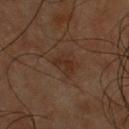  biopsy_status: not biopsied; imaged during a skin examination
  patient:
    sex: male
    age_approx: 60
  automated_metrics:
    area_mm2_approx: 5.0
    eccentricity: 0.65
    shape_asymmetry: 0.4
    border_irregularity_0_10: 3.5
    color_variation_0_10: 2.5
    peripheral_color_asymmetry: 1.0
  image:
    source: total-body photography crop
    field_of_view_mm: 15
  lesion_size:
    long_diameter_mm_approx: 3.0
  lighting: cross-polarized
  site: chest